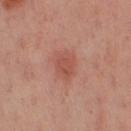Background: A close-up tile cropped from a whole-body skin photograph, about 15 mm across. Located on the left leg. Longest diameter approximately 3.5 mm. Imaged with cross-polarized lighting. A female subject, aged around 40.